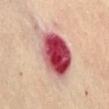Impression:
The lesion was tiled from a total-body skin photograph and was not biopsied.
Clinical summary:
From the abdomen. Cropped from a whole-body photographic skin survey; the tile spans about 15 mm. Measured at roughly 8.5 mm in maximum diameter. A female patient, about 60 years old. The total-body-photography lesion software estimated an area of roughly 27 mm², an outline eccentricity of about 0.75 (0 = round, 1 = elongated), and a shape-asymmetry score of about 0.3 (0 = symmetric).